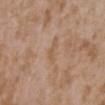Findings:
• biopsy status: catalogued during a skin exam; not biopsied
• site: the chest
• image: ~15 mm tile from a whole-body skin photo
• patient: female, in their mid-30s